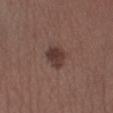The lesion was photographed on a routine skin check and not biopsied; there is no pathology result. This image is a 15 mm lesion crop taken from a total-body photograph. The tile uses white-light illumination. From the left lower leg. The subject is a female aged 53–57.